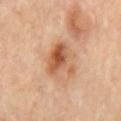No biopsy was performed on this lesion — it was imaged during a full skin examination and was not determined to be concerning. The lesion is on the front of the torso. The subject is a female aged approximately 60. A 15 mm close-up tile from a total-body photography series done for melanoma screening.